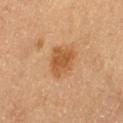follow-up: catalogued during a skin exam; not biopsied | tile lighting: cross-polarized | acquisition: 15 mm crop, total-body photography | lesion diameter: ~4 mm (longest diameter) | TBP lesion metrics: a footprint of about 9 mm² and an outline eccentricity of about 0.5 (0 = round, 1 = elongated); an average lesion color of about L≈47 a*≈21 b*≈34 (CIELAB); a border-irregularity index near 2/10, a color-variation rating of about 2.5/10, and peripheral color asymmetry of about 1 | location: the left thigh | subject: female, approximately 55 years of age.